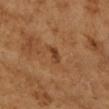Recorded during total-body skin imaging; not selected for excision or biopsy. Cropped from a total-body skin-imaging series; the visible field is about 15 mm. A female subject aged approximately 70. The tile uses cross-polarized illumination. On the left forearm.